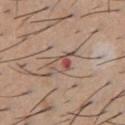No biopsy was performed on this lesion — it was imaged during a full skin examination and was not determined to be concerning. A male subject, roughly 60 years of age. Measured at roughly 4 mm in maximum diameter. Located on the chest. A lesion tile, about 15 mm wide, cut from a 3D total-body photograph. Captured under white-light illumination.